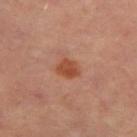<case>
<biopsy_status>not biopsied; imaged during a skin examination</biopsy_status>
<patient>
  <sex>female</sex>
  <age_approx>50</age_approx>
</patient>
<lesion_size>
  <long_diameter_mm_approx>2.5</long_diameter_mm_approx>
</lesion_size>
<site>right thigh</site>
<automated_metrics>
  <vs_skin_darker_L>9.0</vs_skin_darker_L>
  <vs_skin_contrast_norm>8.0</vs_skin_contrast_norm>
  <border_irregularity_0_10>2.0</border_irregularity_0_10>
  <color_variation_0_10>3.0</color_variation_0_10>
  <peripheral_color_asymmetry>1.0</peripheral_color_asymmetry>
  <lesion_detection_confidence_0_100>100</lesion_detection_confidence_0_100>
</automated_metrics>
<image>
  <source>total-body photography crop</source>
  <field_of_view_mm>15</field_of_view_mm>
</image>
</case>